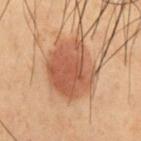notes: catalogued during a skin exam; not biopsied | lesion diameter: ≈6 mm | image: total-body-photography crop, ~15 mm field of view | image-analysis metrics: an outline eccentricity of about 0.6 (0 = round, 1 = elongated) and a symmetry-axis asymmetry near 0.25; a normalized border contrast of about 8; a color-variation rating of about 3/10; a lesion-detection confidence of about 100/100 | subject: male, roughly 55 years of age | lighting: cross-polarized | location: the front of the torso.